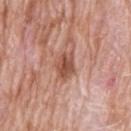{
  "biopsy_status": "not biopsied; imaged during a skin examination",
  "patient": {
    "sex": "male",
    "age_approx": 75
  },
  "site": "upper back",
  "image": {
    "source": "total-body photography crop",
    "field_of_view_mm": 15
  }
}